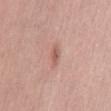Assessment: The lesion was photographed on a routine skin check and not biopsied; there is no pathology result. Background: A male subject, aged around 40. Located on the abdomen. Approximately 2.5 mm at its widest. The tile uses white-light illumination. A roughly 15 mm field-of-view crop from a total-body skin photograph.